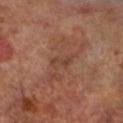Impression: Captured during whole-body skin photography for melanoma surveillance; the lesion was not biopsied. Context: Cropped from a total-body skin-imaging series; the visible field is about 15 mm. On the left lower leg. The patient is a male roughly 70 years of age.